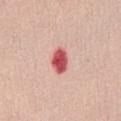Case summary:
* workup — no biopsy performed (imaged during a skin exam)
* site — the abdomen
* patient — female, aged around 60
* image source — 15 mm crop, total-body photography